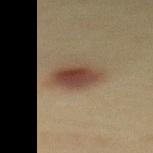The lesion was tiled from a total-body skin photograph and was not biopsied.
The recorded lesion diameter is about 4.5 mm.
A region of skin cropped from a whole-body photographic capture, roughly 15 mm wide.
From the upper back.
The patient is a female aged around 40.